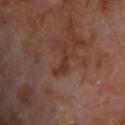– notes — no biopsy performed (imaged during a skin exam)
– automated metrics — a lesion color around L≈33 a*≈21 b*≈26 in CIELAB, a lesion–skin lightness drop of about 6, and a normalized lesion–skin contrast near 7; a color-variation rating of about 0/10 and peripheral color asymmetry of about 0; an automated nevus-likeness rating near 0 out of 100 and lesion-presence confidence of about 100/100
– patient — male, aged around 70
– acquisition — total-body-photography crop, ~15 mm field of view
– size — ~4 mm (longest diameter)
– body site — the back
– tile lighting — cross-polarized